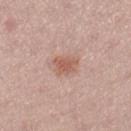follow-up = no biopsy performed (imaged during a skin exam) | lighting = white-light | site = the right lower leg | diameter = ≈3 mm | subject = female, about 25 years old | image = 15 mm crop, total-body photography.